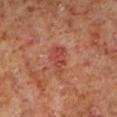Q: Is there a histopathology result?
A: imaged on a skin check; not biopsied
Q: Lesion location?
A: the leg
Q: Illumination type?
A: cross-polarized illumination
Q: How large is the lesion?
A: about 3.5 mm
Q: Automated lesion metrics?
A: an area of roughly 6.5 mm²; a border-irregularity rating of about 3.5/10, internal color variation of about 2.5 on a 0–10 scale, and peripheral color asymmetry of about 1; a classifier nevus-likeness of about 15/100 and a lesion-detection confidence of about 100/100
Q: What are the patient's age and sex?
A: male, aged 58–62
Q: How was this image acquired?
A: ~15 mm tile from a whole-body skin photo